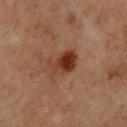Assessment: Part of a total-body skin-imaging series; this lesion was reviewed on a skin check and was not flagged for biopsy. Image and clinical context: Imaged with cross-polarized lighting. The lesion is on the upper back. The subject is a male aged 63 to 67. This image is a 15 mm lesion crop taken from a total-body photograph.